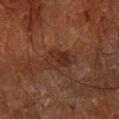{"biopsy_status": "not biopsied; imaged during a skin examination", "image": {"source": "total-body photography crop", "field_of_view_mm": 15}, "patient": {"sex": "male", "age_approx": 60}, "site": "arm"}